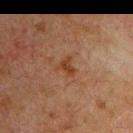Background:
The tile uses cross-polarized illumination. A male subject aged approximately 60. A 15 mm crop from a total-body photograph taken for skin-cancer surveillance. Located on the chest.